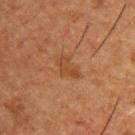Image and clinical context: From the upper back. Imaged with cross-polarized lighting. The lesion's longest dimension is about 3 mm. The subject is a male aged around 50. Cropped from a total-body skin-imaging series; the visible field is about 15 mm. The total-body-photography lesion software estimated a lesion area of about 4.5 mm² and a shape-asymmetry score of about 0.4 (0 = symmetric). The analysis additionally found a mean CIELAB color near L≈35 a*≈19 b*≈30 and about 6 CIELAB-L* units darker than the surrounding skin.